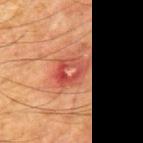No biopsy was performed on this lesion — it was imaged during a full skin examination and was not determined to be concerning.
The lesion is located on the mid back.
Longest diameter approximately 4.5 mm.
Automated tile analysis of the lesion measured an area of roughly 11 mm² and an eccentricity of roughly 0.7. The software also gave roughly 8 lightness units darker than nearby skin. The analysis additionally found a nevus-likeness score of about 0/100 and lesion-presence confidence of about 100/100.
This is a cross-polarized tile.
A male subject, aged 63 to 67.
This image is a 15 mm lesion crop taken from a total-body photograph.